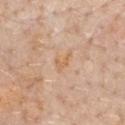workup: total-body-photography surveillance lesion; no biopsy
acquisition: ~15 mm tile from a whole-body skin photo
anatomic site: the chest
diameter: ≈3 mm
image-analysis metrics: a footprint of about 3 mm² and a shape eccentricity near 0.8; a border-irregularity index near 6.5/10, a within-lesion color-variation index near 0/10, and a peripheral color-asymmetry measure near 0; a classifier nevus-likeness of about 0/100
patient: male, in their 60s
tile lighting: white-light